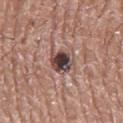workup: imaged on a skin check; not biopsied
anatomic site: the mid back
image: total-body-photography crop, ~15 mm field of view
tile lighting: white-light
patient: male, approximately 70 years of age
lesion diameter: ≈3 mm